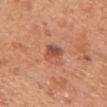Part of a total-body skin-imaging series; this lesion was reviewed on a skin check and was not flagged for biopsy. The patient is a female approximately 50 years of age. The lesion-visualizer software estimated an area of roughly 5 mm², an outline eccentricity of about 0.55 (0 = round, 1 = elongated), and two-axis asymmetry of about 0.25. The software also gave a classifier nevus-likeness of about 70/100 and lesion-presence confidence of about 100/100. Imaged with white-light lighting. From the chest. Approximately 2.5 mm at its widest. A roughly 15 mm field-of-view crop from a total-body skin photograph.